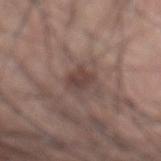The lesion was photographed on a routine skin check and not biopsied; there is no pathology result. A roughly 15 mm field-of-view crop from a total-body skin photograph. A male subject, aged approximately 60. The tile uses white-light illumination. The lesion is located on the abdomen.